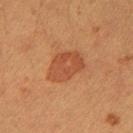workup: catalogued during a skin exam; not biopsied
anatomic site: the right upper arm
diameter: about 4.5 mm
automated metrics: a footprint of about 11 mm² and a shape eccentricity near 0.7; a mean CIELAB color near L≈45 a*≈25 b*≈34, roughly 8 lightness units darker than nearby skin, and a lesion-to-skin contrast of about 6 (normalized; higher = more distinct)
image: 15 mm crop, total-body photography
subject: male, approximately 40 years of age